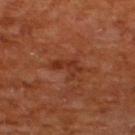Part of a total-body skin-imaging series; this lesion was reviewed on a skin check and was not flagged for biopsy. A 15 mm close-up tile from a total-body photography series done for melanoma screening. On the upper back. The patient is a male aged around 65.